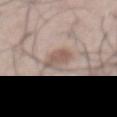A 15 mm close-up extracted from a 3D total-body photography capture. From the abdomen. A male patient, roughly 55 years of age. The total-body-photography lesion software estimated a shape eccentricity near 0.8 and a shape-asymmetry score of about 0.15 (0 = symmetric). It also reported a border-irregularity index near 2/10, a within-lesion color-variation index near 3.5/10, and a peripheral color-asymmetry measure near 1.5.